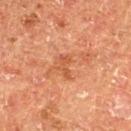Clinical impression:
Part of a total-body skin-imaging series; this lesion was reviewed on a skin check and was not flagged for biopsy.
Acquisition and patient details:
A close-up tile cropped from a whole-body skin photograph, about 15 mm across. The lesion is on the left thigh. The recorded lesion diameter is about 3 mm. The subject is a male aged around 75.